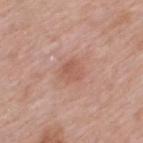Q: Was this lesion biopsied?
A: imaged on a skin check; not biopsied
Q: What kind of image is this?
A: ~15 mm crop, total-body skin-cancer survey
Q: Patient demographics?
A: male, in their mid- to late 50s
Q: What did automated image analysis measure?
A: border irregularity of about 2 on a 0–10 scale and a color-variation rating of about 2.5/10; lesion-presence confidence of about 100/100
Q: Lesion location?
A: the back